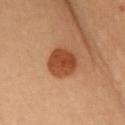<tbp_lesion>
  <biopsy_status>not biopsied; imaged during a skin examination</biopsy_status>
  <automated_metrics>
    <area_mm2_approx>11.0</area_mm2_approx>
    <eccentricity>0.4</eccentricity>
    <vs_skin_darker_L>11.0</vs_skin_darker_L>
    <vs_skin_contrast_norm>9.5</vs_skin_contrast_norm>
    <nevus_likeness_0_100>100</nevus_likeness_0_100>
    <lesion_detection_confidence_0_100>100</lesion_detection_confidence_0_100>
  </automated_metrics>
  <patient>
    <sex>female</sex>
    <age_approx>40</age_approx>
  </patient>
  <image>
    <source>total-body photography crop</source>
    <field_of_view_mm>15</field_of_view_mm>
  </image>
  <site>chest</site>
  <lighting>cross-polarized</lighting>
</tbp_lesion>